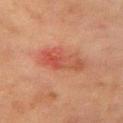Impression: Captured during whole-body skin photography for melanoma surveillance; the lesion was not biopsied. Background: Longest diameter approximately 5 mm. The lesion is located on the left upper arm. Cropped from a total-body skin-imaging series; the visible field is about 15 mm. The tile uses cross-polarized illumination. A female patient aged approximately 80.